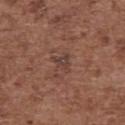follow-up: imaged on a skin check; not biopsied